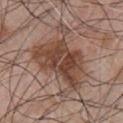Case summary:
– notes · imaged on a skin check; not biopsied
– acquisition · 15 mm crop, total-body photography
– lighting · white-light illumination
– subject · male, aged 48–52
– anatomic site · the chest